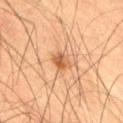Q: Is there a histopathology result?
A: catalogued during a skin exam; not biopsied
Q: How was this image acquired?
A: ~15 mm tile from a whole-body skin photo
Q: Illumination type?
A: cross-polarized illumination
Q: Automated lesion metrics?
A: a footprint of about 3.5 mm² and an outline eccentricity of about 0.7 (0 = round, 1 = elongated); a border-irregularity index near 3/10, internal color variation of about 2.5 on a 0–10 scale, and radial color variation of about 1
Q: How large is the lesion?
A: ≈2.5 mm
Q: Lesion location?
A: the right thigh
Q: Patient demographics?
A: male, aged around 65